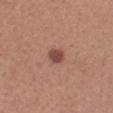Assessment:
Imaged during a routine full-body skin examination; the lesion was not biopsied and no histopathology is available.
Background:
Captured under white-light illumination. A lesion tile, about 15 mm wide, cut from a 3D total-body photograph. A female patient, approximately 35 years of age. The lesion is located on the right forearm. The lesion's longest dimension is about 2.5 mm.